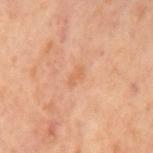biopsy status=total-body-photography surveillance lesion; no biopsy
acquisition=~15 mm tile from a whole-body skin photo
patient=male, aged around 65